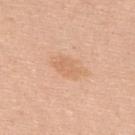Clinical impression:
Recorded during total-body skin imaging; not selected for excision or biopsy.
Acquisition and patient details:
A 15 mm close-up tile from a total-body photography series done for melanoma screening. The subject is a male aged approximately 45. The lesion is on the back. Automated image analysis of the tile measured an eccentricity of roughly 0.85 and a symmetry-axis asymmetry near 0.25. It also reported an average lesion color of about L≈67 a*≈21 b*≈36 (CIELAB), roughly 7 lightness units darker than nearby skin, and a normalized border contrast of about 5. Longest diameter approximately 4 mm.